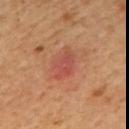Imaged during a routine full-body skin examination; the lesion was not biopsied and no histopathology is available.
The lesion-visualizer software estimated an average lesion color of about L≈49 a*≈29 b*≈30 (CIELAB) and roughly 7 lightness units darker than nearby skin. The software also gave an automated nevus-likeness rating near 25 out of 100.
The patient is a male in their 60s.
About 4 mm across.
On the mid back.
A close-up tile cropped from a whole-body skin photograph, about 15 mm across.